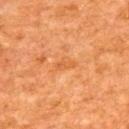Imaged during a routine full-body skin examination; the lesion was not biopsied and no histopathology is available.
The lesion-visualizer software estimated a within-lesion color-variation index near 1.5/10 and a peripheral color-asymmetry measure near 0.5.
A male subject, in their mid- to late 60s.
Located on the upper back.
A region of skin cropped from a whole-body photographic capture, roughly 15 mm wide.
The tile uses cross-polarized illumination.